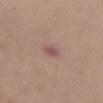<tbp_lesion>
  <lesion_size>
    <long_diameter_mm_approx>2.5</long_diameter_mm_approx>
  </lesion_size>
  <image>
    <source>total-body photography crop</source>
    <field_of_view_mm>15</field_of_view_mm>
  </image>
  <site>abdomen</site>
  <patient>
    <sex>female</sex>
    <age_approx>65</age_approx>
  </patient>
</tbp_lesion>